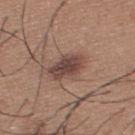<tbp_lesion>
<biopsy_status>not biopsied; imaged during a skin examination</biopsy_status>
<image>
  <source>total-body photography crop</source>
  <field_of_view_mm>15</field_of_view_mm>
</image>
<lesion_size>
  <long_diameter_mm_approx>4.5</long_diameter_mm_approx>
</lesion_size>
<patient>
  <sex>male</sex>
  <age_approx>35</age_approx>
</patient>
<lighting>white-light</lighting>
<site>upper back</site>
<automated_metrics>
  <area_mm2_approx>10.0</area_mm2_approx>
  <eccentricity>0.75</eccentricity>
  <cielab_L>45</cielab_L>
  <cielab_a>18</cielab_a>
  <cielab_b>23</cielab_b>
  <vs_skin_darker_L>11.0</vs_skin_darker_L>
  <vs_skin_contrast_norm>9.0</vs_skin_contrast_norm>
  <border_irregularity_0_10>2.0</border_irregularity_0_10>
  <peripheral_color_asymmetry>1.5</peripheral_color_asymmetry>
  <nevus_likeness_0_100>55</nevus_likeness_0_100>
  <lesion_detection_confidence_0_100>100</lesion_detection_confidence_0_100>
</automated_metrics>
</tbp_lesion>